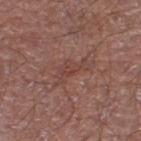follow-up = no biopsy performed (imaged during a skin exam)
lighting = white-light illumination
subject = male, in their 70s
location = the right lower leg
acquisition = ~15 mm crop, total-body skin-cancer survey